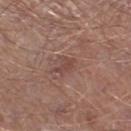The lesion was photographed on a routine skin check and not biopsied; there is no pathology result. On the left lower leg. A 15 mm close-up extracted from a 3D total-body photography capture. A male subject, aged 68 to 72. An algorithmic analysis of the crop reported a lesion area of about 3 mm² and two-axis asymmetry of about 0.4. The analysis additionally found a mean CIELAB color near L≈45 a*≈20 b*≈23. And it measured a classifier nevus-likeness of about 0/100. The lesion's longest dimension is about 2.5 mm.